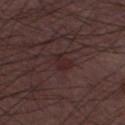Recorded during total-body skin imaging; not selected for excision or biopsy.
Measured at roughly 2.5 mm in maximum diameter.
The lesion is located on the left thigh.
A 15 mm close-up tile from a total-body photography series done for melanoma screening.
A male patient, in their 50s.
This is a white-light tile.